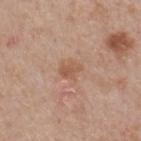follow-up = catalogued during a skin exam; not biopsied.